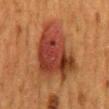On the mid back. The subject is a female roughly 50 years of age. A region of skin cropped from a whole-body photographic capture, roughly 15 mm wide. This is a cross-polarized tile.